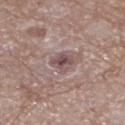Recorded during total-body skin imaging; not selected for excision or biopsy. A close-up tile cropped from a whole-body skin photograph, about 15 mm across. A male patient, aged approximately 85 or older. From the left lower leg. Longest diameter approximately 2.5 mm.